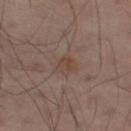{
  "biopsy_status": "not biopsied; imaged during a skin examination",
  "automated_metrics": {
    "cielab_L": 42,
    "cielab_a": 15,
    "cielab_b": 23,
    "vs_skin_darker_L": 5.0,
    "vs_skin_contrast_norm": 5.5,
    "nevus_likeness_0_100": 0,
    "lesion_detection_confidence_0_100": 100
  },
  "patient": {
    "sex": "male",
    "age_approx": 50
  },
  "lesion_size": {
    "long_diameter_mm_approx": 2.5
  },
  "image": {
    "source": "total-body photography crop",
    "field_of_view_mm": 15
  },
  "site": "left leg"
}